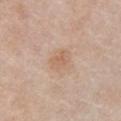Q: Was this lesion biopsied?
A: imaged on a skin check; not biopsied
Q: Where on the body is the lesion?
A: the chest
Q: Illumination type?
A: white-light illumination
Q: What kind of image is this?
A: ~15 mm crop, total-body skin-cancer survey
Q: What are the patient's age and sex?
A: male, aged 73 to 77